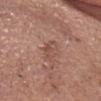Findings:
• follow-up — total-body-photography surveillance lesion; no biopsy
• automated lesion analysis — a lesion area of about 4.5 mm², an eccentricity of roughly 0.85, and two-axis asymmetry of about 0.4; a lesion color around L≈50 a*≈21 b*≈26 in CIELAB, a lesion–skin lightness drop of about 7, and a normalized border contrast of about 5.5; a classifier nevus-likeness of about 0/100 and a lesion-detection confidence of about 100/100
• anatomic site — the head or neck
• diameter — about 3 mm
• imaging modality — ~15 mm tile from a whole-body skin photo
• subject — male, aged approximately 75
• lighting — white-light